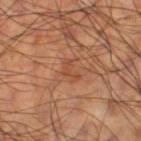The lesion was tiled from a total-body skin photograph and was not biopsied. A 15 mm close-up tile from a total-body photography series done for melanoma screening. A male patient, in their 60s. Located on the left thigh.